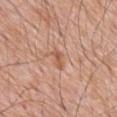workup — no biopsy performed (imaged during a skin exam) | anatomic site — the mid back | image — ~15 mm tile from a whole-body skin photo | subject — male, roughly 80 years of age | lesion size — about 3 mm.